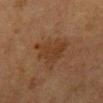Part of a total-body skin-imaging series; this lesion was reviewed on a skin check and was not flagged for biopsy.